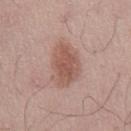No biopsy was performed on this lesion — it was imaged during a full skin examination and was not determined to be concerning. Located on the back. About 5.5 mm across. A lesion tile, about 15 mm wide, cut from a 3D total-body photograph. A male subject, roughly 45 years of age. Automated tile analysis of the lesion measured border irregularity of about 2.5 on a 0–10 scale, a color-variation rating of about 3.5/10, and a peripheral color-asymmetry measure near 1. Imaged with white-light lighting.